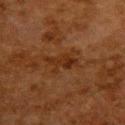{"biopsy_status": "not biopsied; imaged during a skin examination"}